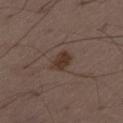workup=catalogued during a skin exam; not biopsied
patient=male, aged approximately 50
imaging modality=~15 mm crop, total-body skin-cancer survey
site=the left thigh